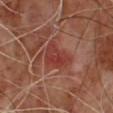Clinical impression:
The lesion was photographed on a routine skin check and not biopsied; there is no pathology result.
Background:
The lesion-visualizer software estimated a footprint of about 8 mm², a shape eccentricity near 0.8, and a symmetry-axis asymmetry near 0.4. It also reported a mean CIELAB color near L≈38 a*≈29 b*≈26, about 7 CIELAB-L* units darker than the surrounding skin, and a normalized border contrast of about 6.5. And it measured a nevus-likeness score of about 0/100 and a lesion-detection confidence of about 100/100. The subject is a male about 65 years old. The recorded lesion diameter is about 4 mm. Cropped from a whole-body photographic skin survey; the tile spans about 15 mm.